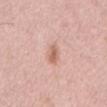Case summary:
* follow-up — imaged on a skin check; not biopsied
* acquisition — ~15 mm tile from a whole-body skin photo
* patient — male, aged 53–57